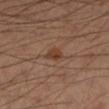Q: Was a biopsy performed?
A: total-body-photography surveillance lesion; no biopsy
Q: Where on the body is the lesion?
A: the right lower leg
Q: What is the imaging modality?
A: ~15 mm crop, total-body skin-cancer survey
Q: What are the patient's age and sex?
A: male, approximately 55 years of age